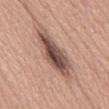A 15 mm crop from a total-body photograph taken for skin-cancer surveillance.
Captured under white-light illumination.
A male patient aged 63–67.
From the abdomen.